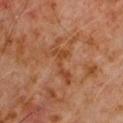acquisition: ~15 mm tile from a whole-body skin photo
site: the chest
subject: male, approximately 60 years of age